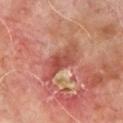Findings:
• histopathology: a squamous cell carcinoma in situ (malignant)